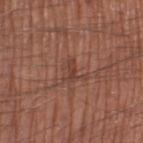Clinical impression:
Recorded during total-body skin imaging; not selected for excision or biopsy.